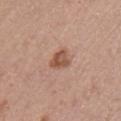<record>
  <biopsy_status>not biopsied; imaged during a skin examination</biopsy_status>
  <image>
    <source>total-body photography crop</source>
    <field_of_view_mm>15</field_of_view_mm>
  </image>
  <site>left upper arm</site>
  <patient>
    <sex>male</sex>
    <age_approx>55</age_approx>
  </patient>
</record>